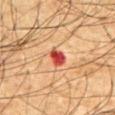workup = total-body-photography surveillance lesion; no biopsy | tile lighting = cross-polarized | site = the front of the torso | acquisition = total-body-photography crop, ~15 mm field of view | subject = male, roughly 65 years of age | size = ≈2.5 mm.